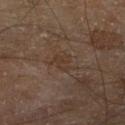| field | value |
|---|---|
| site | the right lower leg |
| lighting | cross-polarized illumination |
| patient | male, aged 68 to 72 |
| image-analysis metrics | two-axis asymmetry of about 0.3; a border-irregularity index near 3.5/10, internal color variation of about 1.5 on a 0–10 scale, and peripheral color asymmetry of about 0.5 |
| imaging modality | total-body-photography crop, ~15 mm field of view |
| size | about 2.5 mm |